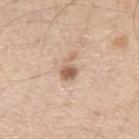Q: Is there a histopathology result?
A: total-body-photography surveillance lesion; no biopsy